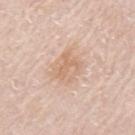biopsy status — imaged on a skin check; not biopsied
image source — ~15 mm crop, total-body skin-cancer survey
location — the left upper arm
subject — male, aged approximately 75
lesion diameter — ≈4 mm